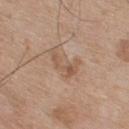A region of skin cropped from a whole-body photographic capture, roughly 15 mm wide.
The lesion is on the back.
A male patient approximately 75 years of age.